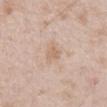| feature | finding |
|---|---|
| biopsy status | imaged on a skin check; not biopsied |
| lesion diameter | about 3 mm |
| image | ~15 mm crop, total-body skin-cancer survey |
| site | the chest |
| patient | male, aged 48–52 |
| illumination | white-light illumination |